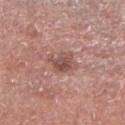Case summary:
• follow-up — no biopsy performed (imaged during a skin exam)
• anatomic site — the right lower leg
• TBP lesion metrics — a lesion-to-skin contrast of about 7 (normalized; higher = more distinct)
• lesion size — ≈3.5 mm
• subject — male, in their mid- to late 50s
• acquisition — total-body-photography crop, ~15 mm field of view